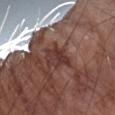workup: no biopsy performed (imaged during a skin exam)
site: the right forearm
diameter: ~4 mm (longest diameter)
illumination: white-light illumination
image source: ~15 mm crop, total-body skin-cancer survey
subject: male, in their mid- to late 70s
automated lesion analysis: internal color variation of about 3.5 on a 0–10 scale and a peripheral color-asymmetry measure near 1.5; a classifier nevus-likeness of about 0/100 and lesion-presence confidence of about 60/100